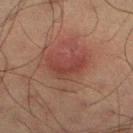Captured during whole-body skin photography for melanoma surveillance; the lesion was not biopsied. Approximately 4.5 mm at its widest. A 15 mm close-up extracted from a 3D total-body photography capture. The lesion-visualizer software estimated an average lesion color of about L≈36 a*≈23 b*≈23 (CIELAB) and a normalized border contrast of about 6. And it measured a within-lesion color-variation index near 2.5/10 and peripheral color asymmetry of about 1. The software also gave a nevus-likeness score of about 100/100 and lesion-presence confidence of about 100/100. This is a cross-polarized tile. A male patient approximately 60 years of age.